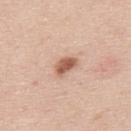Case summary:
• biopsy status · imaged on a skin check; not biopsied
• lesion size · ≈3 mm
• image-analysis metrics · a mean CIELAB color near L≈59 a*≈22 b*≈30, roughly 14 lightness units darker than nearby skin, and a lesion-to-skin contrast of about 9 (normalized; higher = more distinct); a classifier nevus-likeness of about 95/100 and lesion-presence confidence of about 100/100
• patient · male, aged 43–47
• imaging modality · 15 mm crop, total-body photography
• body site · the upper back